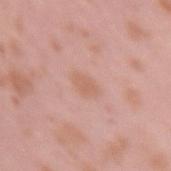| feature | finding |
|---|---|
| biopsy status | no biopsy performed (imaged during a skin exam) |